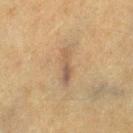Q: Was a biopsy performed?
A: catalogued during a skin exam; not biopsied
Q: Patient demographics?
A: female, about 55 years old
Q: What is the anatomic site?
A: the leg
Q: Illumination type?
A: cross-polarized illumination
Q: What did automated image analysis measure?
A: a footprint of about 4.5 mm² and a shape eccentricity near 0.95; a lesion color around L≈47 a*≈14 b*≈27 in CIELAB, about 8 CIELAB-L* units darker than the surrounding skin, and a normalized lesion–skin contrast near 6.5
Q: What is the imaging modality?
A: ~15 mm tile from a whole-body skin photo
Q: Lesion size?
A: ~4 mm (longest diameter)